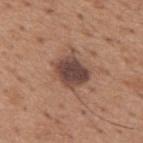biopsy status — catalogued during a skin exam; not biopsied
diameter — ~3.5 mm (longest diameter)
tile lighting — white-light
acquisition — 15 mm crop, total-body photography
automated lesion analysis — a mean CIELAB color near L≈44 a*≈19 b*≈23, a lesion–skin lightness drop of about 14, and a lesion-to-skin contrast of about 11 (normalized; higher = more distinct)
subject — male, aged approximately 65
anatomic site — the upper back